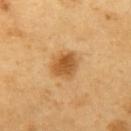Recorded during total-body skin imaging; not selected for excision or biopsy. On the arm. The lesion's longest dimension is about 3.5 mm. The subject is a male in their 60s. Imaged with cross-polarized lighting. A 15 mm close-up tile from a total-body photography series done for melanoma screening. Automated tile analysis of the lesion measured a lesion area of about 8 mm² and a symmetry-axis asymmetry near 0.25. It also reported a mean CIELAB color near L≈55 a*≈22 b*≈43, a lesion–skin lightness drop of about 12, and a lesion-to-skin contrast of about 8.5 (normalized; higher = more distinct).